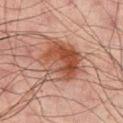Case summary:
– notes — no biopsy performed (imaged during a skin exam)
– lesion diameter — about 5.5 mm
– automated metrics — a lesion area of about 20 mm² and a shape-asymmetry score of about 0.25 (0 = symmetric)
– patient — male, in their mid-60s
– body site — the back
– tile lighting — cross-polarized
– acquisition — ~15 mm crop, total-body skin-cancer survey